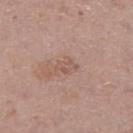Q: Is there a histopathology result?
A: catalogued during a skin exam; not biopsied
Q: Lesion location?
A: the right lower leg
Q: Automated lesion metrics?
A: an area of roughly 3 mm², an eccentricity of roughly 0.85, and a symmetry-axis asymmetry near 0.4; roughly 7 lightness units darker than nearby skin and a normalized lesion–skin contrast near 5; border irregularity of about 4.5 on a 0–10 scale and peripheral color asymmetry of about 0.5; an automated nevus-likeness rating near 0 out of 100 and a lesion-detection confidence of about 100/100
Q: What kind of image is this?
A: total-body-photography crop, ~15 mm field of view
Q: Who is the patient?
A: female, aged around 50